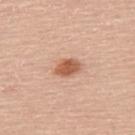Notes:
* notes — imaged on a skin check; not biopsied
* automated metrics — a classifier nevus-likeness of about 95/100
* anatomic site — the upper back
* illumination — white-light illumination
* lesion size — ≈3 mm
* patient — male, aged around 60
* image source — total-body-photography crop, ~15 mm field of view